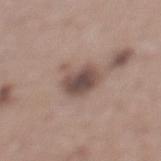No biopsy was performed on this lesion — it was imaged during a full skin examination and was not determined to be concerning. Located on the left lower leg. Cropped from a total-body skin-imaging series; the visible field is about 15 mm. The patient is a male in their mid-60s. Longest diameter approximately 4.5 mm.